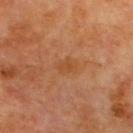Captured during whole-body skin photography for melanoma surveillance; the lesion was not biopsied. A male subject aged approximately 60. The lesion is on the upper back. A 15 mm crop from a total-body photograph taken for skin-cancer surveillance.